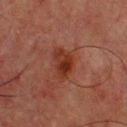This is a cross-polarized tile.
A 15 mm crop from a total-body photograph taken for skin-cancer surveillance.
A male subject roughly 65 years of age.
From the upper back.